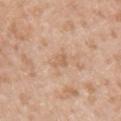Assessment: The lesion was photographed on a routine skin check and not biopsied; there is no pathology result. Image and clinical context: A male patient approximately 35 years of age. The lesion is located on the left upper arm. The total-body-photography lesion software estimated an eccentricity of roughly 0.85 and a shape-asymmetry score of about 0.3 (0 = symmetric). Imaged with white-light lighting. A 15 mm close-up extracted from a 3D total-body photography capture. About 2.5 mm across.